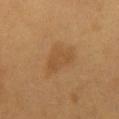Acquisition and patient details: The tile uses cross-polarized illumination. The patient is a male about 60 years old. Located on the chest. Longest diameter approximately 5 mm. Automated tile analysis of the lesion measured a lesion area of about 9 mm² and an outline eccentricity of about 0.85 (0 = round, 1 = elongated). And it measured a lesion–skin lightness drop of about 6 and a lesion-to-skin contrast of about 5 (normalized; higher = more distinct). The software also gave a border-irregularity index near 4/10, a within-lesion color-variation index near 2/10, and peripheral color asymmetry of about 0.5. And it measured a nevus-likeness score of about 30/100. A lesion tile, about 15 mm wide, cut from a 3D total-body photograph.